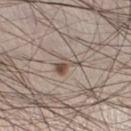Background: From the left lower leg. Longest diameter approximately 3 mm. Automated tile analysis of the lesion measured a border-irregularity rating of about 5.5/10, a color-variation rating of about 5.5/10, and radial color variation of about 2. And it measured an automated nevus-likeness rating near 75 out of 100 and a detector confidence of about 95 out of 100 that the crop contains a lesion. A male patient, in their mid-30s. Imaged with white-light lighting. A 15 mm crop from a total-body photograph taken for skin-cancer surveillance.